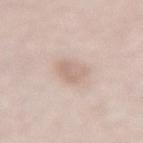biopsy_status: not biopsied; imaged during a skin examination
lesion_size:
  long_diameter_mm_approx: 3.0
automated_metrics:
  area_mm2_approx: 6.5
  eccentricity: 0.6
  border_irregularity_0_10: 2.5
  color_variation_0_10: 2.5
  peripheral_color_asymmetry: 1.0
  nevus_likeness_0_100: 15
  lesion_detection_confidence_0_100: 100
site: lower back
lighting: white-light
image:
  source: total-body photography crop
  field_of_view_mm: 15
patient:
  sex: female
  age_approx: 65